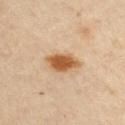The lesion was photographed on a routine skin check and not biopsied; there is no pathology result.
Captured under cross-polarized illumination.
The total-body-photography lesion software estimated a lesion area of about 8.5 mm² and a symmetry-axis asymmetry near 0.2. And it measured a mean CIELAB color near L≈49 a*≈18 b*≈35, roughly 12 lightness units darker than nearby skin, and a normalized lesion–skin contrast near 10.5. The analysis additionally found a color-variation rating of about 3/10 and radial color variation of about 0.5.
The subject is a female approximately 45 years of age.
The lesion is located on the chest.
Cropped from a whole-body photographic skin survey; the tile spans about 15 mm.